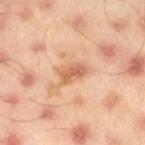No biopsy was performed on this lesion — it was imaged during a full skin examination and was not determined to be concerning. The patient is a male aged approximately 45. From the right thigh. A 15 mm close-up tile from a total-body photography series done for melanoma screening. An algorithmic analysis of the crop reported a lesion area of about 5.5 mm², an outline eccentricity of about 0.8 (0 = round, 1 = elongated), and a shape-asymmetry score of about 0.25 (0 = symmetric). The software also gave an automated nevus-likeness rating near 0 out of 100 and a detector confidence of about 100 out of 100 that the crop contains a lesion.